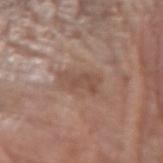biopsy_status: not biopsied; imaged during a skin examination
lesion_size:
  long_diameter_mm_approx: 4.0
image:
  source: total-body photography crop
  field_of_view_mm: 15
patient:
  sex: female
  age_approx: 80
site: arm
lighting: white-light